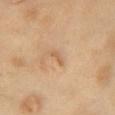Q: How was this image acquired?
A: total-body-photography crop, ~15 mm field of view
Q: What are the patient's age and sex?
A: female, roughly 55 years of age
Q: Automated lesion metrics?
A: a lesion area of about 1.5 mm², a shape eccentricity near 0.95, and a shape-asymmetry score of about 0.55 (0 = symmetric); roughly 8 lightness units darker than nearby skin and a normalized lesion–skin contrast near 5.5; a nevus-likeness score of about 0/100 and a lesion-detection confidence of about 100/100
Q: Lesion location?
A: the chest